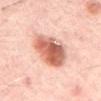Impression: The lesion was photographed on a routine skin check and not biopsied; there is no pathology result.